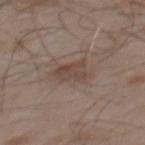| feature | finding |
|---|---|
| follow-up | imaged on a skin check; not biopsied |
| lesion size | ≈3.5 mm |
| image source | ~15 mm crop, total-body skin-cancer survey |
| patient | male, aged around 55 |
| lighting | white-light illumination |
| location | the mid back |